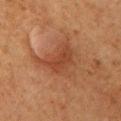A female patient approximately 60 years of age.
The lesion's longest dimension is about 6 mm.
The lesion is on the upper back.
Captured under cross-polarized illumination.
A 15 mm crop from a total-body photograph taken for skin-cancer surveillance.